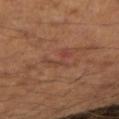Captured during whole-body skin photography for melanoma surveillance; the lesion was not biopsied. Measured at roughly 3.5 mm in maximum diameter. A 15 mm close-up extracted from a 3D total-body photography capture. Automated tile analysis of the lesion measured a footprint of about 4.5 mm², an outline eccentricity of about 0.75 (0 = round, 1 = elongated), and a shape-asymmetry score of about 0.7 (0 = symmetric). And it measured an average lesion color of about L≈41 a*≈23 b*≈27 (CIELAB), a lesion–skin lightness drop of about 5, and a normalized lesion–skin contrast near 5. It also reported a classifier nevus-likeness of about 0/100 and lesion-presence confidence of about 100/100. Imaged with cross-polarized lighting. Located on the right forearm. A male patient, aged 43–47.